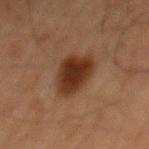Clinical impression: This lesion was catalogued during total-body skin photography and was not selected for biopsy.